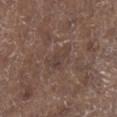* notes — catalogued during a skin exam; not biopsied
* subject — male, approximately 70 years of age
* lighting — white-light illumination
* acquisition — total-body-photography crop, ~15 mm field of view
* anatomic site — the leg
* lesion size — ≈3.5 mm